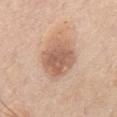Impression: Recorded during total-body skin imaging; not selected for excision or biopsy. Background: The recorded lesion diameter is about 5.5 mm. From the chest. Imaged with white-light lighting. A 15 mm close-up tile from a total-body photography series done for melanoma screening. A male subject, approximately 75 years of age. The total-body-photography lesion software estimated an average lesion color of about L≈60 a*≈20 b*≈30 (CIELAB), a lesion–skin lightness drop of about 12, and a normalized lesion–skin contrast near 7.5. The software also gave a border-irregularity index near 1.5/10, a within-lesion color-variation index near 4/10, and peripheral color asymmetry of about 1.5. The software also gave a classifier nevus-likeness of about 30/100.